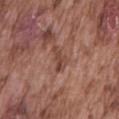{"biopsy_status": "not biopsied; imaged during a skin examination", "automated_metrics": {"area_mm2_approx": 3.0, "eccentricity": 0.9, "cielab_L": 44, "cielab_a": 22, "cielab_b": 26, "vs_skin_darker_L": 9.0, "border_irregularity_0_10": 5.5}, "image": {"source": "total-body photography crop", "field_of_view_mm": 15}, "site": "mid back", "patient": {"sex": "male", "age_approx": 75}}